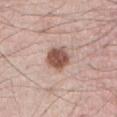<lesion>
  <biopsy_status>not biopsied; imaged during a skin examination</biopsy_status>
  <lighting>white-light</lighting>
  <lesion_size>
    <long_diameter_mm_approx>3.0</long_diameter_mm_approx>
  </lesion_size>
  <site>abdomen</site>
  <image>
    <source>total-body photography crop</source>
    <field_of_view_mm>15</field_of_view_mm>
  </image>
  <patient>
    <sex>male</sex>
    <age_approx>70</age_approx>
  </patient>
</lesion>